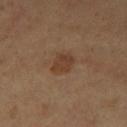workup=imaged on a skin check; not biopsied | image=total-body-photography crop, ~15 mm field of view | anatomic site=the leg | TBP lesion metrics=an area of roughly 5.5 mm², a shape eccentricity near 0.5, and a symmetry-axis asymmetry near 0.3 | illumination=cross-polarized illumination | diameter=~3 mm (longest diameter) | subject=female, roughly 65 years of age.